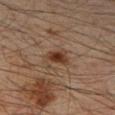The lesion was tiled from a total-body skin photograph and was not biopsied. Cropped from a whole-body photographic skin survey; the tile spans about 15 mm. On the right forearm. A male patient, in their mid- to late 40s.